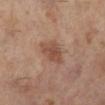The lesion was tiled from a total-body skin photograph and was not biopsied.
A female subject aged around 60.
The lesion is on the leg.
Captured under cross-polarized illumination.
Measured at roughly 4 mm in maximum diameter.
This image is a 15 mm lesion crop taken from a total-body photograph.